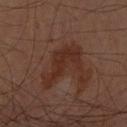Assessment:
The lesion was photographed on a routine skin check and not biopsied; there is no pathology result.
Acquisition and patient details:
The recorded lesion diameter is about 5.5 mm. The patient is a male aged 58 to 62. The tile uses cross-polarized illumination. The lesion is on the left forearm. A region of skin cropped from a whole-body photographic capture, roughly 15 mm wide. Automated tile analysis of the lesion measured an average lesion color of about L≈25 a*≈18 b*≈22 (CIELAB), a lesion–skin lightness drop of about 6, and a lesion-to-skin contrast of about 7 (normalized; higher = more distinct). And it measured a classifier nevus-likeness of about 0/100 and lesion-presence confidence of about 100/100.